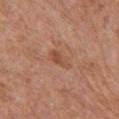Clinical impression:
This lesion was catalogued during total-body skin photography and was not selected for biopsy.